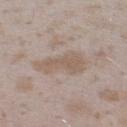Assessment:
No biopsy was performed on this lesion — it was imaged during a full skin examination and was not determined to be concerning.
Context:
A female subject aged 23 to 27. Located on the left lower leg. This is a white-light tile. Automated tile analysis of the lesion measured a footprint of about 10 mm² and two-axis asymmetry of about 0.4. The analysis additionally found internal color variation of about 1.5 on a 0–10 scale and peripheral color asymmetry of about 0.5. And it measured a nevus-likeness score of about 0/100 and lesion-presence confidence of about 65/100. This image is a 15 mm lesion crop taken from a total-body photograph.